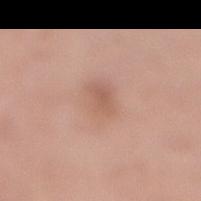Q: Is there a histopathology result?
A: total-body-photography surveillance lesion; no biopsy
Q: What is the imaging modality?
A: total-body-photography crop, ~15 mm field of view
Q: Where on the body is the lesion?
A: the right lower leg
Q: What did automated image analysis measure?
A: a border-irregularity rating of about 1.5/10, a within-lesion color-variation index near 4/10, and a peripheral color-asymmetry measure near 1.5
Q: What lighting was used for the tile?
A: white-light
Q: How large is the lesion?
A: ~4 mm (longest diameter)
Q: What are the patient's age and sex?
A: female, aged 53 to 57